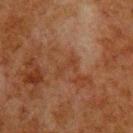Impression:
This lesion was catalogued during total-body skin photography and was not selected for biopsy.
Image and clinical context:
A male patient about 80 years old. A lesion tile, about 15 mm wide, cut from a 3D total-body photograph. The lesion is located on the upper back. An algorithmic analysis of the crop reported a footprint of about 3 mm², a shape eccentricity near 0.95, and a symmetry-axis asymmetry near 0.3. It also reported a border-irregularity index near 4.5/10. The analysis additionally found an automated nevus-likeness rating near 0 out of 100. This is a cross-polarized tile.